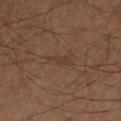Impression:
The lesion was tiled from a total-body skin photograph and was not biopsied.
Acquisition and patient details:
The total-body-photography lesion software estimated internal color variation of about 0.5 on a 0–10 scale and peripheral color asymmetry of about 0. The analysis additionally found a classifier nevus-likeness of about 0/100 and lesion-presence confidence of about 95/100. About 3 mm across. A 15 mm close-up tile from a total-body photography series done for melanoma screening. This is a cross-polarized tile. Located on the left lower leg. A male subject aged approximately 60.